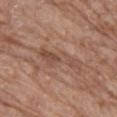| key | value |
|---|---|
| notes | total-body-photography surveillance lesion; no biopsy |
| image source | ~15 mm crop, total-body skin-cancer survey |
| patient | male, approximately 80 years of age |
| size | ~6 mm (longest diameter) |
| anatomic site | the left thigh |